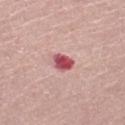This lesion was catalogued during total-body skin photography and was not selected for biopsy. A 15 mm close-up extracted from a 3D total-body photography capture. The patient is a female roughly 60 years of age. The lesion is located on the left thigh. The tile uses white-light illumination. About 2.5 mm across. Automated tile analysis of the lesion measured a border-irregularity index near 2.5/10 and a peripheral color-asymmetry measure near 2. And it measured an automated nevus-likeness rating near 0 out of 100 and a detector confidence of about 100 out of 100 that the crop contains a lesion.